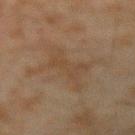<tbp_lesion>
  <biopsy_status>not biopsied; imaged during a skin examination</biopsy_status>
  <patient>
    <sex>male</sex>
    <age_approx>45</age_approx>
  </patient>
  <site>right forearm</site>
  <lesion_size>
    <long_diameter_mm_approx>5.5</long_diameter_mm_approx>
  </lesion_size>
  <image>
    <source>total-body photography crop</source>
    <field_of_view_mm>15</field_of_view_mm>
  </image>
  <lighting>cross-polarized</lighting>
</tbp_lesion>